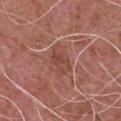{"biopsy_status": "not biopsied; imaged during a skin examination", "patient": {"sex": "male", "age_approx": 75}, "site": "chest", "image": {"source": "total-body photography crop", "field_of_view_mm": 15}}